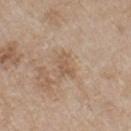| field | value |
|---|---|
| follow-up | imaged on a skin check; not biopsied |
| imaging modality | 15 mm crop, total-body photography |
| patient | male, about 65 years old |
| anatomic site | the chest |